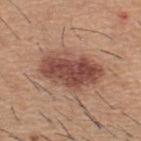follow-up: imaged on a skin check; not biopsied
lesion size: ~7 mm (longest diameter)
tile lighting: white-light illumination
imaging modality: 15 mm crop, total-body photography
anatomic site: the upper back
patient: male, about 60 years old